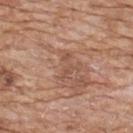{
  "biopsy_status": "not biopsied; imaged during a skin examination",
  "image": {
    "source": "total-body photography crop",
    "field_of_view_mm": 15
  },
  "patient": {
    "sex": "male",
    "age_approx": 60
  },
  "site": "chest"
}